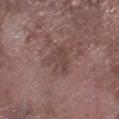Impression:
This lesion was catalogued during total-body skin photography and was not selected for biopsy.
Background:
The recorded lesion diameter is about 3.5 mm. Automated tile analysis of the lesion measured a lesion color around L≈43 a*≈18 b*≈20 in CIELAB and a lesion–skin lightness drop of about 6. And it measured a classifier nevus-likeness of about 0/100 and lesion-presence confidence of about 100/100. A male patient in their mid-70s. A lesion tile, about 15 mm wide, cut from a 3D total-body photograph. The lesion is on the right lower leg.